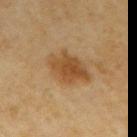biopsy status — no biopsy performed (imaged during a skin exam) | subject — female, in their mid- to late 50s | image — 15 mm crop, total-body photography | TBP lesion metrics — an average lesion color of about L≈42 a*≈17 b*≈34 (CIELAB), a lesion–skin lightness drop of about 9, and a lesion-to-skin contrast of about 8 (normalized; higher = more distinct); border irregularity of about 3 on a 0–10 scale, a within-lesion color-variation index near 3.5/10, and peripheral color asymmetry of about 1; a classifier nevus-likeness of about 90/100 and a detector confidence of about 100 out of 100 that the crop contains a lesion | tile lighting — cross-polarized | lesion size — ≈5.5 mm | location — the arm.